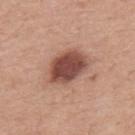Part of a total-body skin-imaging series; this lesion was reviewed on a skin check and was not flagged for biopsy. About 5 mm across. An algorithmic analysis of the crop reported a mean CIELAB color near L≈47 a*≈24 b*≈26, roughly 17 lightness units darker than nearby skin, and a normalized lesion–skin contrast near 11.5. The software also gave border irregularity of about 2 on a 0–10 scale, a within-lesion color-variation index near 4/10, and peripheral color asymmetry of about 1. And it measured an automated nevus-likeness rating near 75 out of 100 and lesion-presence confidence of about 100/100. The patient is a male aged 53 to 57. Imaged with white-light lighting. A 15 mm crop from a total-body photograph taken for skin-cancer surveillance. The lesion is on the upper back.